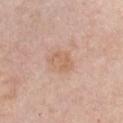The lesion was tiled from a total-body skin photograph and was not biopsied.
The lesion is on the chest.
The subject is a female in their mid-60s.
A lesion tile, about 15 mm wide, cut from a 3D total-body photograph.